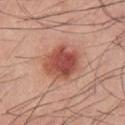Longest diameter approximately 4.5 mm. An algorithmic analysis of the crop reported a footprint of about 15 mm², an eccentricity of roughly 0.45, and two-axis asymmetry of about 0.15. Imaged with white-light lighting. A lesion tile, about 15 mm wide, cut from a 3D total-body photograph. The subject is a male in their mid- to late 40s. The lesion is on the chest.